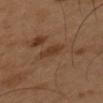Impression: No biopsy was performed on this lesion — it was imaged during a full skin examination and was not determined to be concerning. Clinical summary: A 15 mm close-up extracted from a 3D total-body photography capture. Located on the left upper arm. The subject is a male aged 48–52.